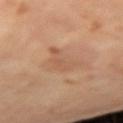Clinical impression:
Imaged during a routine full-body skin examination; the lesion was not biopsied and no histopathology is available.